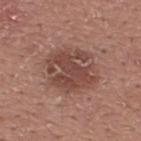Q: Was this lesion biopsied?
A: imaged on a skin check; not biopsied
Q: What kind of image is this?
A: total-body-photography crop, ~15 mm field of view
Q: Lesion location?
A: the upper back
Q: What is the lesion's diameter?
A: ~5 mm (longest diameter)
Q: Who is the patient?
A: male, in their 20s
Q: Automated lesion metrics?
A: a footprint of about 16 mm², an eccentricity of roughly 0.45, and a symmetry-axis asymmetry near 0.3; a lesion color around L≈44 a*≈22 b*≈24 in CIELAB, about 10 CIELAB-L* units darker than the surrounding skin, and a normalized border contrast of about 8; a classifier nevus-likeness of about 15/100 and lesion-presence confidence of about 100/100